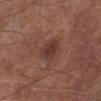Notes:
– patient · male, aged 53–57
– location · the left lower leg
– tile lighting · white-light
– diameter · ≈3 mm
– imaging modality · 15 mm crop, total-body photography